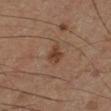Findings:
* biopsy status · imaged on a skin check; not biopsied
* diameter · about 3 mm
* patient · male, about 60 years old
* tile lighting · cross-polarized
* site · the right lower leg
* image source · ~15 mm crop, total-body skin-cancer survey